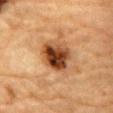Part of a total-body skin-imaging series; this lesion was reviewed on a skin check and was not flagged for biopsy. A 15 mm crop from a total-body photograph taken for skin-cancer surveillance. Measured at roughly 4.5 mm in maximum diameter. Located on the chest. A male subject, in their mid-80s or older. Automated tile analysis of the lesion measured a lesion color around L≈39 a*≈22 b*≈33 in CIELAB, roughly 17 lightness units darker than nearby skin, and a normalized border contrast of about 13. This is a cross-polarized tile.